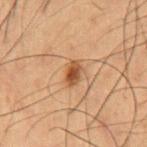Context: The tile uses cross-polarized illumination. On the chest. This image is a 15 mm lesion crop taken from a total-body photograph. The patient is a male aged 48 to 52.